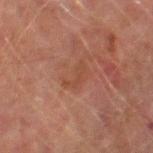Assessment: This lesion was catalogued during total-body skin photography and was not selected for biopsy. Context: A lesion tile, about 15 mm wide, cut from a 3D total-body photograph. A male patient in their mid- to late 70s. From the right thigh.